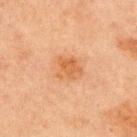The lesion was photographed on a routine skin check and not biopsied; there is no pathology result. The lesion is located on the chest. A 15 mm crop from a total-body photograph taken for skin-cancer surveillance. A male subject aged 63–67. Approximately 3.5 mm at its widest.